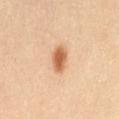Imaged during a routine full-body skin examination; the lesion was not biopsied and no histopathology is available. A roughly 15 mm field-of-view crop from a total-body skin photograph. A female subject aged 53 to 57. The recorded lesion diameter is about 3 mm. Located on the left thigh. The tile uses cross-polarized illumination.